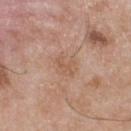Captured during whole-body skin photography for melanoma surveillance; the lesion was not biopsied. Cropped from a whole-body photographic skin survey; the tile spans about 15 mm. The patient is a male about 50 years old. The lesion is located on the front of the torso. Captured under white-light illumination.